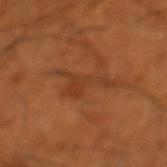Assessment: Part of a total-body skin-imaging series; this lesion was reviewed on a skin check and was not flagged for biopsy. Context: Imaged with cross-polarized lighting. A lesion tile, about 15 mm wide, cut from a 3D total-body photograph. Measured at roughly 7 mm in maximum diameter. The subject is a male aged 63 to 67. Located on the left lower leg.